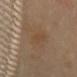The lesion was tiled from a total-body skin photograph and was not biopsied. The tile uses cross-polarized illumination. The lesion is located on the front of the torso. About 4 mm across. Automated tile analysis of the lesion measured an area of roughly 4 mm² and a shape eccentricity near 0.95. It also reported a mean CIELAB color near L≈45 a*≈15 b*≈31, about 5 CIELAB-L* units darker than the surrounding skin, and a normalized border contrast of about 5. And it measured a nevus-likeness score of about 0/100 and a detector confidence of about 100 out of 100 that the crop contains a lesion. The subject is a female aged around 60. This image is a 15 mm lesion crop taken from a total-body photograph.